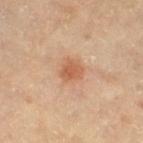notes: catalogued during a skin exam; not biopsied
tile lighting: cross-polarized illumination
site: the right thigh
automated lesion analysis: an average lesion color of about L≈59 a*≈24 b*≈36 (CIELAB)
image source: ~15 mm crop, total-body skin-cancer survey
subject: male, aged around 65
size: about 2.5 mm